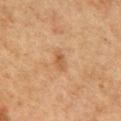{"biopsy_status": "not biopsied; imaged during a skin examination", "patient": {"sex": "male", "age_approx": 75}, "site": "front of the torso", "lesion_size": {"long_diameter_mm_approx": 2.5}, "image": {"source": "total-body photography crop", "field_of_view_mm": 15}, "automated_metrics": {"cielab_L": 45, "cielab_a": 18, "cielab_b": 31, "vs_skin_darker_L": 7.0, "vs_skin_contrast_norm": 6.0, "border_irregularity_0_10": 3.0, "color_variation_0_10": 2.5, "peripheral_color_asymmetry": 1.0}, "lighting": "cross-polarized"}